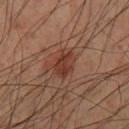Q: Is there a histopathology result?
A: no biopsy performed (imaged during a skin exam)
Q: How was this image acquired?
A: ~15 mm tile from a whole-body skin photo
Q: Patient demographics?
A: male, roughly 50 years of age
Q: Lesion size?
A: ≈3.5 mm
Q: Automated lesion metrics?
A: an eccentricity of roughly 0.7 and two-axis asymmetry of about 0.15; an average lesion color of about L≈37 a*≈23 b*≈27 (CIELAB), roughly 9 lightness units darker than nearby skin, and a normalized border contrast of about 7.5; a classifier nevus-likeness of about 60/100 and a detector confidence of about 100 out of 100 that the crop contains a lesion
Q: What is the anatomic site?
A: the left lower leg
Q: How was the tile lit?
A: cross-polarized illumination